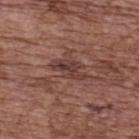Part of a total-body skin-imaging series; this lesion was reviewed on a skin check and was not flagged for biopsy. On the upper back. Cropped from a total-body skin-imaging series; the visible field is about 15 mm. A female subject, roughly 65 years of age.